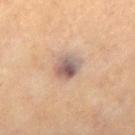The lesion was photographed on a routine skin check and not biopsied; there is no pathology result. This image is a 15 mm lesion crop taken from a total-body photograph. An algorithmic analysis of the crop reported a lesion color around L≈42 a*≈13 b*≈18 in CIELAB and a normalized lesion–skin contrast near 9.5. The analysis additionally found a classifier nevus-likeness of about 5/100 and lesion-presence confidence of about 100/100. On the mid back. A female subject aged 78 to 82. About 3.5 mm across.